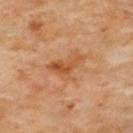biopsy_status: not biopsied; imaged during a skin examination
lighting: cross-polarized
automated_metrics:
  eccentricity: 0.75
  shape_asymmetry: 0.55
  nevus_likeness_0_100: 0
  lesion_detection_confidence_0_100: 100
lesion_size:
  long_diameter_mm_approx: 5.0
site: back
image:
  source: total-body photography crop
  field_of_view_mm: 15